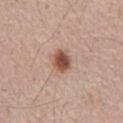Captured during whole-body skin photography for melanoma surveillance; the lesion was not biopsied.